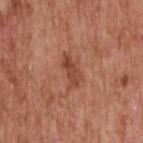No biopsy was performed on this lesion — it was imaged during a full skin examination and was not determined to be concerning. The lesion-visualizer software estimated a footprint of about 5 mm² and two-axis asymmetry of about 0.35. The software also gave a border-irregularity rating of about 4/10 and a within-lesion color-variation index near 3/10. The analysis additionally found a nevus-likeness score of about 10/100 and a lesion-detection confidence of about 100/100. Approximately 4 mm at its widest. A male patient in their 60s. A region of skin cropped from a whole-body photographic capture, roughly 15 mm wide. The lesion is on the back. The tile uses white-light illumination.